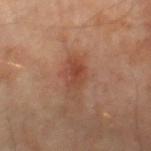Located on the right lower leg.
Approximately 3.5 mm at its widest.
A patient aged approximately 55.
A lesion tile, about 15 mm wide, cut from a 3D total-body photograph.
An algorithmic analysis of the crop reported roughly 8 lightness units darker than nearby skin and a normalized lesion–skin contrast near 6.5. And it measured an automated nevus-likeness rating near 45 out of 100 and lesion-presence confidence of about 100/100.
The tile uses cross-polarized illumination.